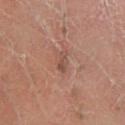| field | value |
|---|---|
| follow-up | catalogued during a skin exam; not biopsied |
| illumination | cross-polarized illumination |
| patient | female, aged 48 to 52 |
| image | ~15 mm crop, total-body skin-cancer survey |
| diameter | about 2.5 mm |